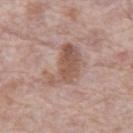The lesion was tiled from a total-body skin photograph and was not biopsied.
A male subject, in their 70s.
Located on the front of the torso.
A close-up tile cropped from a whole-body skin photograph, about 15 mm across.